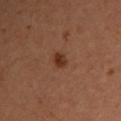follow-up: imaged on a skin check; not biopsied
acquisition: total-body-photography crop, ~15 mm field of view
TBP lesion metrics: border irregularity of about 2.5 on a 0–10 scale, internal color variation of about 2.5 on a 0–10 scale, and a peripheral color-asymmetry measure near 1; a nevus-likeness score of about 95/100 and lesion-presence confidence of about 100/100
location: the upper back
patient: male, in their mid-30s
lesion diameter: ≈2 mm
lighting: cross-polarized